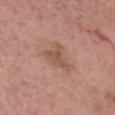– follow-up · no biopsy performed (imaged during a skin exam)
– image · 15 mm crop, total-body photography
– anatomic site · the head or neck
– patient · male, about 80 years old